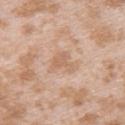<case>
<biopsy_status>not biopsied; imaged during a skin examination</biopsy_status>
<lesion_size>
  <long_diameter_mm_approx>2.5</long_diameter_mm_approx>
</lesion_size>
<site>upper back</site>
<patient>
  <sex>female</sex>
  <age_approx>25</age_approx>
</patient>
<image>
  <source>total-body photography crop</source>
  <field_of_view_mm>15</field_of_view_mm>
</image>
<lighting>white-light</lighting>
</case>